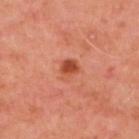Q: Was a biopsy performed?
A: imaged on a skin check; not biopsied
Q: Illumination type?
A: cross-polarized
Q: What is the imaging modality?
A: 15 mm crop, total-body photography
Q: Lesion location?
A: the back
Q: Patient demographics?
A: male, approximately 50 years of age